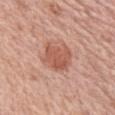Imaged during a routine full-body skin examination; the lesion was not biopsied and no histopathology is available.
The lesion's longest dimension is about 4 mm.
Captured under white-light illumination.
A female subject, in their mid- to late 60s.
A roughly 15 mm field-of-view crop from a total-body skin photograph.
Automated image analysis of the tile measured a border-irregularity index near 3/10, a within-lesion color-variation index near 3/10, and a peripheral color-asymmetry measure near 1. It also reported an automated nevus-likeness rating near 90 out of 100 and a lesion-detection confidence of about 100/100.
From the left upper arm.